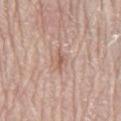notes: total-body-photography surveillance lesion; no biopsy
subject: male, about 80 years old
image source: total-body-photography crop, ~15 mm field of view
location: the mid back